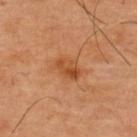Case summary:
– biopsy status · total-body-photography surveillance lesion; no biopsy
– body site · the upper back
– acquisition · ~15 mm tile from a whole-body skin photo
– patient · male, in their mid- to late 40s